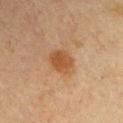This lesion was catalogued during total-body skin photography and was not selected for biopsy. The lesion is located on the right upper arm. A region of skin cropped from a whole-body photographic capture, roughly 15 mm wide. Imaged with cross-polarized lighting. The lesion's longest dimension is about 3 mm. The subject is a male in their mid-60s.